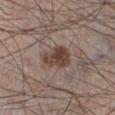Clinical impression: The lesion was photographed on a routine skin check and not biopsied; there is no pathology result. Clinical summary: The patient is a male aged 58–62. A 15 mm crop from a total-body photograph taken for skin-cancer surveillance. The lesion is located on the leg. Captured under white-light illumination.